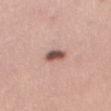No biopsy was performed on this lesion — it was imaged during a full skin examination and was not determined to be concerning.
Located on the right thigh.
A female subject aged approximately 35.
The lesion-visualizer software estimated an area of roughly 5 mm². And it measured an average lesion color of about L≈54 a*≈20 b*≈24 (CIELAB), a lesion–skin lightness drop of about 16, and a lesion-to-skin contrast of about 10.5 (normalized; higher = more distinct). The analysis additionally found a classifier nevus-likeness of about 90/100 and a lesion-detection confidence of about 100/100.
The recorded lesion diameter is about 3 mm.
Captured under white-light illumination.
A 15 mm close-up tile from a total-body photography series done for melanoma screening.